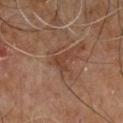Imaged during a routine full-body skin examination; the lesion was not biopsied and no histopathology is available.
Cropped from a whole-body photographic skin survey; the tile spans about 15 mm.
A male subject, aged around 60.
From the abdomen.